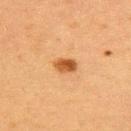notes: imaged on a skin check; not biopsied
location: the upper back
image: 15 mm crop, total-body photography
patient: female, aged 38 to 42